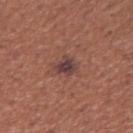biopsy status: total-body-photography surveillance lesion; no biopsy | site: the right upper arm | subject: male, in their mid- to late 40s | tile lighting: white-light illumination | acquisition: ~15 mm crop, total-body skin-cancer survey | automated metrics: a footprint of about 5.5 mm² and two-axis asymmetry of about 0.3; an average lesion color of about L≈41 a*≈20 b*≈21 (CIELAB), a lesion–skin lightness drop of about 9, and a normalized lesion–skin contrast near 9; lesion-presence confidence of about 100/100 | lesion diameter: ~3 mm (longest diameter).